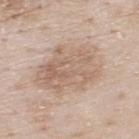{
  "biopsy_status": "not biopsied; imaged during a skin examination",
  "lighting": "white-light",
  "automated_metrics": {
    "border_irregularity_0_10": 2.5,
    "color_variation_0_10": 4.5,
    "peripheral_color_asymmetry": 1.5,
    "nevus_likeness_0_100": 5,
    "lesion_detection_confidence_0_100": 100
  },
  "image": {
    "source": "total-body photography crop",
    "field_of_view_mm": 15
  },
  "site": "upper back",
  "lesion_size": {
    "long_diameter_mm_approx": 8.0
  },
  "patient": {
    "sex": "male",
    "age_approx": 80
  }
}